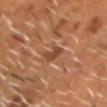Notes:
– workup · total-body-photography surveillance lesion; no biopsy
– acquisition · 15 mm crop, total-body photography
– lesion size · ≈2.5 mm
– lighting · cross-polarized illumination
– anatomic site · the head or neck
– subject · male, in their mid- to late 50s